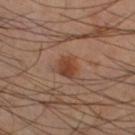<record>
  <biopsy_status>not biopsied; imaged during a skin examination</biopsy_status>
  <site>leg</site>
  <automated_metrics>
    <area_mm2_approx>5.0</area_mm2_approx>
    <eccentricity>0.7</eccentricity>
    <shape_asymmetry>0.2</shape_asymmetry>
    <vs_skin_darker_L>9.0</vs_skin_darker_L>
    <vs_skin_contrast_norm>8.5</vs_skin_contrast_norm>
    <nevus_likeness_0_100>90</nevus_likeness_0_100>
    <lesion_detection_confidence_0_100>100</lesion_detection_confidence_0_100>
  </automated_metrics>
  <patient>
    <sex>male</sex>
    <age_approx>40</age_approx>
  </patient>
  <image>
    <source>total-body photography crop</source>
    <field_of_view_mm>15</field_of_view_mm>
  </image>
  <lighting>cross-polarized</lighting>
  <lesion_size>
    <long_diameter_mm_approx>2.5</long_diameter_mm_approx>
  </lesion_size>
</record>